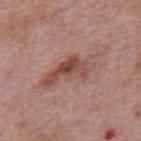| feature | finding |
|---|---|
| follow-up | imaged on a skin check; not biopsied |
| automated metrics | an area of roughly 12 mm² and an outline eccentricity of about 0.9 (0 = round, 1 = elongated) |
| subject | male, aged around 75 |
| image source | ~15 mm tile from a whole-body skin photo |
| illumination | white-light illumination |
| size | ≈6.5 mm |
| anatomic site | the back |